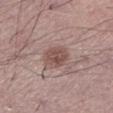Imaged during a routine full-body skin examination; the lesion was not biopsied and no histopathology is available. The patient is a male approximately 60 years of age. About 3 mm across. A roughly 15 mm field-of-view crop from a total-body skin photograph. The lesion is on the left lower leg.